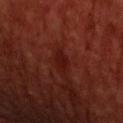Notes:
- patient: male, aged 58 to 62
- site: the head or neck
- image-analysis metrics: an outline eccentricity of about 0.8 (0 = round, 1 = elongated) and two-axis asymmetry of about 0.25; a border-irregularity index near 2/10, a within-lesion color-variation index near 1.5/10, and peripheral color asymmetry of about 0.5
- illumination: cross-polarized illumination
- size: ~2.5 mm (longest diameter)
- image source: total-body-photography crop, ~15 mm field of view